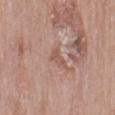Clinical impression:
The lesion was tiled from a total-body skin photograph and was not biopsied.
Background:
The lesion is located on the mid back. The lesion's longest dimension is about 2.5 mm. A close-up tile cropped from a whole-body skin photograph, about 15 mm across. Imaged with white-light lighting. A female patient aged 58 to 62.